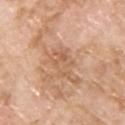A male subject aged 58 to 62.
The tile uses white-light illumination.
Cropped from a total-body skin-imaging series; the visible field is about 15 mm.
Measured at roughly 4.5 mm in maximum diameter.
The lesion is located on the back.